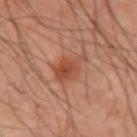patient: male, roughly 40 years of age | illumination: cross-polarized | image: 15 mm crop, total-body photography | TBP lesion metrics: an outline eccentricity of about 0.8 (0 = round, 1 = elongated) and two-axis asymmetry of about 0.4; a mean CIELAB color near L≈47 a*≈26 b*≈32, about 9 CIELAB-L* units darker than the surrounding skin, and a normalized lesion–skin contrast near 7; a border-irregularity rating of about 4.5/10, internal color variation of about 4 on a 0–10 scale, and peripheral color asymmetry of about 1 | diameter: ~4.5 mm (longest diameter) | location: the left upper arm.